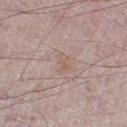  biopsy_status: not biopsied; imaged during a skin examination
  patient:
    sex: male
    age_approx: 75
  lighting: white-light
  site: left thigh
  image:
    source: total-body photography crop
    field_of_view_mm: 15
  lesion_size:
    long_diameter_mm_approx: 2.5